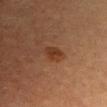notes: no biopsy performed (imaged during a skin exam)
lighting: cross-polarized illumination
body site: the head or neck
TBP lesion metrics: a lesion area of about 4 mm², a shape eccentricity near 0.7, and a shape-asymmetry score of about 0.3 (0 = symmetric); a lesion color around L≈32 a*≈21 b*≈29 in CIELAB, a lesion–skin lightness drop of about 7, and a normalized border contrast of about 7; border irregularity of about 2.5 on a 0–10 scale, a within-lesion color-variation index near 2/10, and a peripheral color-asymmetry measure near 0.5; a classifier nevus-likeness of about 70/100 and a detector confidence of about 100 out of 100 that the crop contains a lesion
acquisition: ~15 mm tile from a whole-body skin photo
subject: male, aged around 35